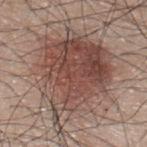follow-up = total-body-photography surveillance lesion; no biopsy | imaging modality = ~15 mm crop, total-body skin-cancer survey | automated lesion analysis = a mean CIELAB color near L≈47 a*≈19 b*≈23, a lesion–skin lightness drop of about 12, and a normalized border contrast of about 9; a lesion-detection confidence of about 100/100 | anatomic site = the upper back | lesion diameter = ≈12 mm | subject = male, roughly 45 years of age | tile lighting = white-light illumination.